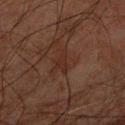Clinical impression:
This lesion was catalogued during total-body skin photography and was not selected for biopsy.
Context:
On the right forearm. A 15 mm close-up tile from a total-body photography series done for melanoma screening. Imaged with cross-polarized lighting. The patient is a male in their 80s.